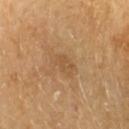| key | value |
|---|---|
| notes | imaged on a skin check; not biopsied |
| site | the arm |
| subject | female, in their 70s |
| illumination | cross-polarized illumination |
| image-analysis metrics | a detector confidence of about 100 out of 100 that the crop contains a lesion |
| image source | ~15 mm tile from a whole-body skin photo |